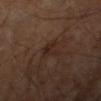Clinical impression: The lesion was photographed on a routine skin check and not biopsied; there is no pathology result. Clinical summary: A lesion tile, about 15 mm wide, cut from a 3D total-body photograph. From the left arm. The patient is a male aged 58–62. The recorded lesion diameter is about 3 mm.